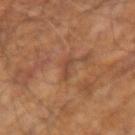Captured during whole-body skin photography for melanoma surveillance; the lesion was not biopsied. The recorded lesion diameter is about 3 mm. On the right upper arm. The patient is a male about 65 years old. Cropped from a whole-body photographic skin survey; the tile spans about 15 mm. The tile uses cross-polarized illumination.